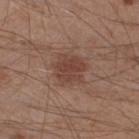Impression: Imaged during a routine full-body skin examination; the lesion was not biopsied and no histopathology is available. Background: A 15 mm close-up extracted from a 3D total-body photography capture. On the left lower leg. The subject is a male roughly 30 years of age. About 4 mm across.